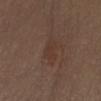biopsy status=no biopsy performed (imaged during a skin exam)
lesion size=~4.5 mm (longest diameter)
TBP lesion metrics=a footprint of about 6 mm²; a mean CIELAB color near L≈35 a*≈17 b*≈24 and a normalized border contrast of about 5
subject=male, aged 68 to 72
lighting=white-light illumination
body site=the abdomen
acquisition=~15 mm tile from a whole-body skin photo